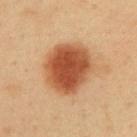{
  "biopsy_status": "not biopsied; imaged during a skin examination",
  "site": "abdomen",
  "patient": {
    "sex": "male",
    "age_approx": 50
  },
  "image": {
    "source": "total-body photography crop",
    "field_of_view_mm": 15
  }
}